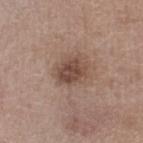Findings:
– notes · imaged on a skin check; not biopsied
– site · the right lower leg
– image · 15 mm crop, total-body photography
– tile lighting · white-light illumination
– patient · male, roughly 50 years of age
– image-analysis metrics · an automated nevus-likeness rating near 15 out of 100 and a lesion-detection confidence of about 100/100